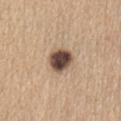Notes:
* notes · no biopsy performed (imaged during a skin exam)
* subject · female, aged 58 to 62
* anatomic site · the abdomen
* image · total-body-photography crop, ~15 mm field of view